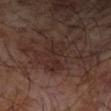Imaged during a routine full-body skin examination; the lesion was not biopsied and no histopathology is available.
The lesion is on the right forearm.
Longest diameter approximately 4.5 mm.
The subject is a male roughly 65 years of age.
This is a cross-polarized tile.
A region of skin cropped from a whole-body photographic capture, roughly 15 mm wide.